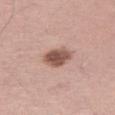<tbp_lesion>
<biopsy_status>not biopsied; imaged during a skin examination</biopsy_status>
</tbp_lesion>